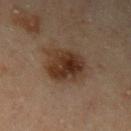Q: What kind of image is this?
A: ~15 mm tile from a whole-body skin photo
Q: What are the patient's age and sex?
A: male, approximately 45 years of age
Q: What is the anatomic site?
A: the left upper arm